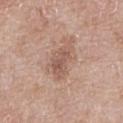workup = no biopsy performed (imaged during a skin exam)
tile lighting = white-light illumination
image source = total-body-photography crop, ~15 mm field of view
lesion diameter = ~4.5 mm (longest diameter)
subject = male, aged around 70
anatomic site = the chest